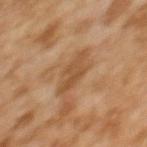<case>
  <biopsy_status>not biopsied; imaged during a skin examination</biopsy_status>
  <image>
    <source>total-body photography crop</source>
    <field_of_view_mm>15</field_of_view_mm>
  </image>
  <lighting>cross-polarized</lighting>
  <site>upper back</site>
  <automated_metrics>
    <area_mm2_approx>6.5</area_mm2_approx>
    <eccentricity>0.85</eccentricity>
    <shape_asymmetry>0.3</shape_asymmetry>
    <vs_skin_darker_L>8.0</vs_skin_darker_L>
    <vs_skin_contrast_norm>6.5</vs_skin_contrast_norm>
    <border_irregularity_0_10>4.0</border_irregularity_0_10>
    <color_variation_0_10>2.0</color_variation_0_10>
    <peripheral_color_asymmetry>0.5</peripheral_color_asymmetry>
    <nevus_likeness_0_100>0</nevus_likeness_0_100>
    <lesion_detection_confidence_0_100>95</lesion_detection_confidence_0_100>
  </automated_metrics>
  <patient>
    <sex>female</sex>
    <age_approx>60</age_approx>
  </patient>
  <lesion_size>
    <long_diameter_mm_approx>4.0</long_diameter_mm_approx>
  </lesion_size>
</case>